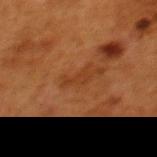| key | value |
|---|---|
| biopsy status | no biopsy performed (imaged during a skin exam) |
| location | the upper back |
| tile lighting | cross-polarized |
| subject | female, roughly 50 years of age |
| acquisition | ~15 mm crop, total-body skin-cancer survey |
| lesion size | about 3 mm |